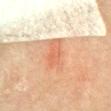{
  "biopsy_status": "not biopsied; imaged during a skin examination",
  "patient": {
    "sex": "male",
    "age_approx": 70
  },
  "lighting": "cross-polarized",
  "lesion_size": {
    "long_diameter_mm_approx": 5.0
  },
  "site": "chest",
  "image": {
    "source": "total-body photography crop",
    "field_of_view_mm": 15
  }
}